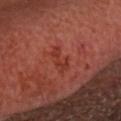The lesion was tiled from a total-body skin photograph and was not biopsied. Longest diameter approximately 3 mm. Captured under cross-polarized illumination. The lesion is located on the head or neck. A region of skin cropped from a whole-body photographic capture, roughly 15 mm wide. The patient is a male aged 63–67.